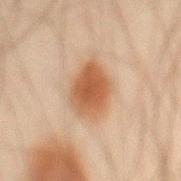- notes — no biopsy performed (imaged during a skin exam)
- illumination — cross-polarized illumination
- site — the abdomen
- imaging modality — ~15 mm tile from a whole-body skin photo
- subject — male, about 45 years old
- size — about 5 mm